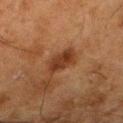Notes:
* notes · catalogued during a skin exam; not biopsied
* TBP lesion metrics · a lesion area of about 7.5 mm², a shape eccentricity near 0.75, and two-axis asymmetry of about 0.25; about 9 CIELAB-L* units darker than the surrounding skin and a lesion-to-skin contrast of about 9 (normalized; higher = more distinct); a border-irregularity rating of about 3/10 and a within-lesion color-variation index near 2.5/10
* site · the right thigh
* image · ~15 mm crop, total-body skin-cancer survey
* lighting · cross-polarized illumination
* subject · male, roughly 80 years of age
* size · ≈3.5 mm